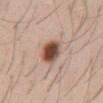Clinical summary:
The lesion is on the abdomen. A male subject, aged 28–32. A lesion tile, about 15 mm wide, cut from a 3D total-body photograph. Imaged with white-light lighting. Approximately 3.5 mm at its widest.